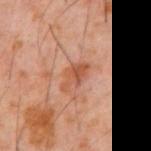The lesion was tiled from a total-body skin photograph and was not biopsied.
Located on the abdomen.
The subject is a male aged around 60.
The lesion's longest dimension is about 3.5 mm.
A close-up tile cropped from a whole-body skin photograph, about 15 mm across.